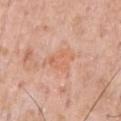No biopsy was performed on this lesion — it was imaged during a full skin examination and was not determined to be concerning. Approximately 3.5 mm at its widest. Imaged with white-light lighting. The lesion is on the front of the torso. Cropped from a total-body skin-imaging series; the visible field is about 15 mm. The subject is a male approximately 60 years of age.